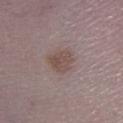The lesion was tiled from a total-body skin photograph and was not biopsied.
A close-up tile cropped from a whole-body skin photograph, about 15 mm across.
From the left lower leg.
Captured under white-light illumination.
The subject is a male aged 48 to 52.